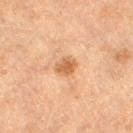follow-up: catalogued during a skin exam; not biopsied